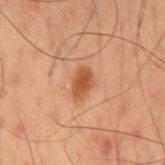Image and clinical context:
The lesion is located on the mid back. A roughly 15 mm field-of-view crop from a total-body skin photograph. The recorded lesion diameter is about 3 mm. Imaged with cross-polarized lighting. The lesion-visualizer software estimated a lesion area of about 5 mm², an eccentricity of roughly 0.8, and a shape-asymmetry score of about 0.2 (0 = symmetric). The analysis additionally found a lesion–skin lightness drop of about 9. The subject is a male aged around 50.